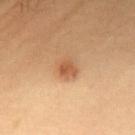Assessment:
The lesion was tiled from a total-body skin photograph and was not biopsied.
Image and clinical context:
The lesion's longest dimension is about 3 mm. Located on the upper back. A 15 mm close-up extracted from a 3D total-body photography capture. The tile uses cross-polarized illumination. A male subject, about 40 years old.